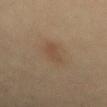- notes · total-body-photography surveillance lesion; no biopsy
- imaging modality · total-body-photography crop, ~15 mm field of view
- patient · male, about 40 years old
- anatomic site · the mid back